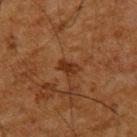  biopsy_status: not biopsied; imaged during a skin examination
  site: upper back
  image:
    source: total-body photography crop
    field_of_view_mm: 15
  patient:
    sex: male
    age_approx: 65